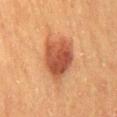Captured under cross-polarized illumination.
An algorithmic analysis of the crop reported an area of roughly 18 mm². And it measured a mean CIELAB color near L≈48 a*≈26 b*≈34, about 13 CIELAB-L* units darker than the surrounding skin, and a normalized lesion–skin contrast near 9.5. The software also gave a nevus-likeness score of about 100/100 and a detector confidence of about 100 out of 100 that the crop contains a lesion.
A 15 mm close-up extracted from a 3D total-body photography capture.
Longest diameter approximately 5.5 mm.
A male patient, approximately 60 years of age.
On the mid back.